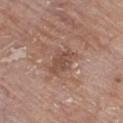<case>
<biopsy_status>not biopsied; imaged during a skin examination</biopsy_status>
<site>chest</site>
<image>
  <source>total-body photography crop</source>
  <field_of_view_mm>15</field_of_view_mm>
</image>
<automated_metrics>
  <cielab_L>49</cielab_L>
  <cielab_a>20</cielab_a>
  <cielab_b>26</cielab_b>
  <vs_skin_darker_L>8.0</vs_skin_darker_L>
  <vs_skin_contrast_norm>6.0</vs_skin_contrast_norm>
  <nevus_likeness_0_100>0</nevus_likeness_0_100>
</automated_metrics>
<patient>
  <sex>male</sex>
  <age_approx>80</age_approx>
</patient>
<lesion_size>
  <long_diameter_mm_approx>3.5</long_diameter_mm_approx>
</lesion_size>
</case>